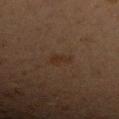Clinical impression: The lesion was tiled from a total-body skin photograph and was not biopsied. Acquisition and patient details: Imaged with cross-polarized lighting. Measured at roughly 2.5 mm in maximum diameter. A region of skin cropped from a whole-body photographic capture, roughly 15 mm wide. The total-body-photography lesion software estimated internal color variation of about 0.5 on a 0–10 scale. It also reported a classifier nevus-likeness of about 20/100 and lesion-presence confidence of about 100/100. Located on the right forearm. A female patient aged approximately 40.